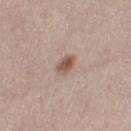Assessment:
No biopsy was performed on this lesion — it was imaged during a full skin examination and was not determined to be concerning.
Context:
A roughly 15 mm field-of-view crop from a total-body skin photograph. A female patient, aged around 40. The lesion's longest dimension is about 2.5 mm. An algorithmic analysis of the crop reported a lesion area of about 4 mm² and a shape eccentricity near 0.75. And it measured a border-irregularity index near 2/10, a color-variation rating of about 4.5/10, and a peripheral color-asymmetry measure near 1.5. The software also gave a nevus-likeness score of about 95/100 and lesion-presence confidence of about 100/100. Imaged with white-light lighting. The lesion is on the right thigh.